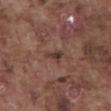site: abdomen
patient:
  sex: male
  age_approx: 75
lighting: white-light
image:
  source: total-body photography crop
  field_of_view_mm: 15
lesion_size:
  long_diameter_mm_approx: 2.5